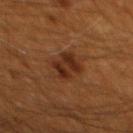Part of a total-body skin-imaging series; this lesion was reviewed on a skin check and was not flagged for biopsy. The total-body-photography lesion software estimated a mean CIELAB color near L≈27 a*≈21 b*≈28 and roughly 8 lightness units darker than nearby skin. The analysis additionally found an automated nevus-likeness rating near 70 out of 100 and a detector confidence of about 100 out of 100 that the crop contains a lesion. The lesion is on the mid back. The patient is a male in their 60s. A lesion tile, about 15 mm wide, cut from a 3D total-body photograph. Imaged with cross-polarized lighting. Approximately 4.5 mm at its widest.